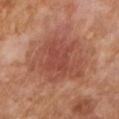Part of a total-body skin-imaging series; this lesion was reviewed on a skin check and was not flagged for biopsy.
On the right forearm.
The patient is a female roughly 50 years of age.
A 15 mm close-up tile from a total-body photography series done for melanoma screening.